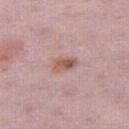workup: catalogued during a skin exam; not biopsied | image source: total-body-photography crop, ~15 mm field of view | subject: female, aged 28–32 | automated metrics: an area of roughly 4.5 mm², an outline eccentricity of about 0.85 (0 = round, 1 = elongated), and a shape-asymmetry score of about 0.25 (0 = symmetric); roughly 10 lightness units darker than nearby skin and a lesion-to-skin contrast of about 8 (normalized; higher = more distinct); a nevus-likeness score of about 80/100 and a detector confidence of about 100 out of 100 that the crop contains a lesion | size: ~3 mm (longest diameter) | site: the right thigh.